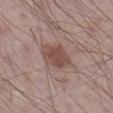Impression:
No biopsy was performed on this lesion — it was imaged during a full skin examination and was not determined to be concerning.
Acquisition and patient details:
About 4 mm across. A male patient, aged around 60. On the right lower leg. A roughly 15 mm field-of-view crop from a total-body skin photograph. The tile uses white-light illumination.